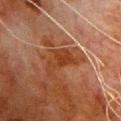Q: Was this lesion biopsied?
A: total-body-photography surveillance lesion; no biopsy
Q: How was the tile lit?
A: cross-polarized
Q: What did automated image analysis measure?
A: a lesion–skin lightness drop of about 6 and a normalized lesion–skin contrast near 7.5; a nevus-likeness score of about 0/100 and lesion-presence confidence of about 95/100
Q: How was this image acquired?
A: ~15 mm tile from a whole-body skin photo
Q: Lesion size?
A: ~4.5 mm (longest diameter)
Q: Who is the patient?
A: male, aged around 80
Q: Lesion location?
A: the front of the torso